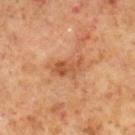Q: What did automated image analysis measure?
A: an area of roughly 7.5 mm² and a shape-asymmetry score of about 0.3 (0 = symmetric); an average lesion color of about L≈51 a*≈24 b*≈35 (CIELAB), roughly 9 lightness units darker than nearby skin, and a normalized lesion–skin contrast near 6.5; border irregularity of about 4 on a 0–10 scale, a color-variation rating of about 4.5/10, and radial color variation of about 1.5; a detector confidence of about 100 out of 100 that the crop contains a lesion
Q: What kind of image is this?
A: ~15 mm tile from a whole-body skin photo
Q: Who is the patient?
A: male, in their 60s
Q: What is the anatomic site?
A: the right lower leg
Q: How large is the lesion?
A: about 4.5 mm